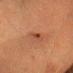<tbp_lesion>
<biopsy_status>not biopsied; imaged during a skin examination</biopsy_status>
<image>
  <source>total-body photography crop</source>
  <field_of_view_mm>15</field_of_view_mm>
</image>
<patient>
  <sex>female</sex>
  <age_approx>55</age_approx>
</patient>
<site>head or neck</site>
</tbp_lesion>